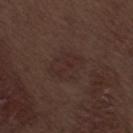Assessment:
The lesion was tiled from a total-body skin photograph and was not biopsied.
Acquisition and patient details:
The patient is a male roughly 70 years of age. Cropped from a whole-body photographic skin survey; the tile spans about 15 mm. From the leg. Captured under white-light illumination.